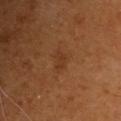No biopsy was performed on this lesion — it was imaged during a full skin examination and was not determined to be concerning.
A lesion tile, about 15 mm wide, cut from a 3D total-body photograph.
The subject is a male roughly 60 years of age.
The lesion is located on the chest.
This is a cross-polarized tile.
The lesion's longest dimension is about 2.5 mm.
The total-body-photography lesion software estimated a mean CIELAB color near L≈33 a*≈21 b*≈32, about 5 CIELAB-L* units darker than the surrounding skin, and a normalized lesion–skin contrast near 5. It also reported a border-irregularity rating of about 3/10 and internal color variation of about 1 on a 0–10 scale. The software also gave a nevus-likeness score of about 0/100 and lesion-presence confidence of about 100/100.